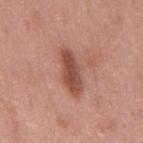biopsy status: total-body-photography surveillance lesion; no biopsy
size: ~5.5 mm (longest diameter)
location: the mid back
acquisition: ~15 mm tile from a whole-body skin photo
subject: male, aged 48 to 52
lighting: white-light illumination
image-analysis metrics: an area of roughly 11 mm², an outline eccentricity of about 0.9 (0 = round, 1 = elongated), and two-axis asymmetry of about 0.15